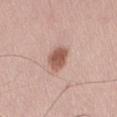lighting: white-light
lesion_size:
  long_diameter_mm_approx: 3.0
site: abdomen
patient:
  sex: male
  age_approx: 70
image:
  source: total-body photography crop
  field_of_view_mm: 15